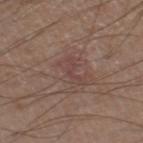Q: Is there a histopathology result?
A: catalogued during a skin exam; not biopsied
Q: What is the anatomic site?
A: the right lower leg
Q: Who is the patient?
A: male, in their 20s
Q: What is the imaging modality?
A: 15 mm crop, total-body photography
Q: Automated lesion metrics?
A: an area of roughly 5.5 mm² and an eccentricity of roughly 0.85; a mean CIELAB color near L≈44 a*≈18 b*≈21, about 5 CIELAB-L* units darker than the surrounding skin, and a normalized border contrast of about 4.5; a border-irregularity index near 6/10 and radial color variation of about 0.5; a detector confidence of about 95 out of 100 that the crop contains a lesion
Q: Illumination type?
A: white-light illumination
Q: How large is the lesion?
A: about 4 mm